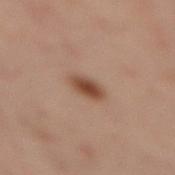Assessment:
The lesion was photographed on a routine skin check and not biopsied; there is no pathology result.
Context:
Longest diameter approximately 3 mm. An algorithmic analysis of the crop reported an area of roughly 5 mm², an eccentricity of roughly 0.8, and a shape-asymmetry score of about 0.15 (0 = symmetric). The software also gave a lesion color around L≈38 a*≈17 b*≈24 in CIELAB, about 10 CIELAB-L* units darker than the surrounding skin, and a lesion-to-skin contrast of about 9 (normalized; higher = more distinct). It also reported an automated nevus-likeness rating near 100 out of 100. A close-up tile cropped from a whole-body skin photograph, about 15 mm across. Imaged with cross-polarized lighting. A female patient aged 53 to 57. Located on the mid back.